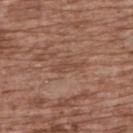About 3.5 mm across.
A 15 mm close-up tile from a total-body photography series done for melanoma screening.
A female patient in their mid- to late 70s.
From the upper back.
Automated tile analysis of the lesion measured a border-irregularity index near 6.5/10, a color-variation rating of about 0/10, and radial color variation of about 0.